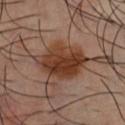Q: Is there a histopathology result?
A: total-body-photography surveillance lesion; no biopsy
Q: Where on the body is the lesion?
A: the chest
Q: What is the imaging modality?
A: ~15 mm crop, total-body skin-cancer survey
Q: What is the lesion's diameter?
A: ≈6 mm
Q: What did automated image analysis measure?
A: a mean CIELAB color near L≈36 a*≈21 b*≈29, about 12 CIELAB-L* units darker than the surrounding skin, and a lesion-to-skin contrast of about 11.5 (normalized; higher = more distinct); border irregularity of about 2 on a 0–10 scale and a color-variation rating of about 5.5/10; a classifier nevus-likeness of about 90/100 and a lesion-detection confidence of about 100/100
Q: Patient demographics?
A: male, aged 38–42